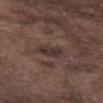{
  "biopsy_status": "not biopsied; imaged during a skin examination"
}